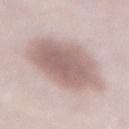Q: Was this lesion biopsied?
A: imaged on a skin check; not biopsied
Q: What is the imaging modality?
A: total-body-photography crop, ~15 mm field of view
Q: What is the anatomic site?
A: the mid back
Q: What did automated image analysis measure?
A: an area of roughly 35 mm², a shape eccentricity near 0.5, and two-axis asymmetry of about 0.15; a lesion color around L≈62 a*≈17 b*≈20 in CIELAB and a normalized lesion–skin contrast near 8; a classifier nevus-likeness of about 50/100 and a detector confidence of about 80 out of 100 that the crop contains a lesion
Q: Lesion size?
A: ~7.5 mm (longest diameter)
Q: Who is the patient?
A: male, roughly 55 years of age